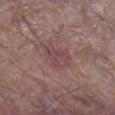Assessment:
No biopsy was performed on this lesion — it was imaged during a full skin examination and was not determined to be concerning.
Image and clinical context:
Captured under white-light illumination. The patient is a male about 80 years old. Located on the right lower leg. This image is a 15 mm lesion crop taken from a total-body photograph. The recorded lesion diameter is about 4 mm. An algorithmic analysis of the crop reported a footprint of about 7.5 mm², an outline eccentricity of about 0.8 (0 = round, 1 = elongated), and two-axis asymmetry of about 0.3. It also reported a border-irregularity rating of about 3/10, a color-variation rating of about 3.5/10, and peripheral color asymmetry of about 1. And it measured an automated nevus-likeness rating near 0 out of 100 and lesion-presence confidence of about 95/100.